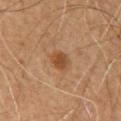This lesion was catalogued during total-body skin photography and was not selected for biopsy.
The patient is a male in their mid- to late 60s.
Located on the chest.
A 15 mm close-up tile from a total-body photography series done for melanoma screening.